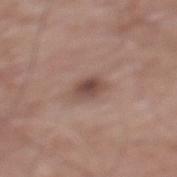The lesion was photographed on a routine skin check and not biopsied; there is no pathology result. The lesion-visualizer software estimated an area of roughly 5 mm². It also reported about 11 CIELAB-L* units darker than the surrounding skin and a normalized lesion–skin contrast near 8. It also reported a border-irregularity index near 1.5/10 and a within-lesion color-variation index near 4/10. Cropped from a whole-body photographic skin survey; the tile spans about 15 mm. Longest diameter approximately 3 mm. A male patient, aged around 60. On the mid back. The tile uses white-light illumination.